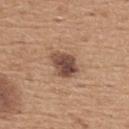Imaged during a routine full-body skin examination; the lesion was not biopsied and no histopathology is available. Measured at roughly 4 mm in maximum diameter. The tile uses white-light illumination. The total-body-photography lesion software estimated a lesion area of about 8.5 mm². And it measured a border-irregularity index near 2.5/10, internal color variation of about 6 on a 0–10 scale, and peripheral color asymmetry of about 2. A 15 mm close-up extracted from a 3D total-body photography capture. A male patient, approximately 65 years of age. From the upper back.